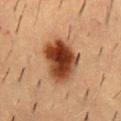Part of a total-body skin-imaging series; this lesion was reviewed on a skin check and was not flagged for biopsy. Automated image analysis of the tile measured an average lesion color of about L≈37 a*≈22 b*≈31 (CIELAB), about 17 CIELAB-L* units darker than the surrounding skin, and a normalized lesion–skin contrast near 13.5. The software also gave a detector confidence of about 100 out of 100 that the crop contains a lesion. Located on the back. This image is a 15 mm lesion crop taken from a total-body photograph. Longest diameter approximately 6 mm. The tile uses cross-polarized illumination. The subject is a male roughly 55 years of age.